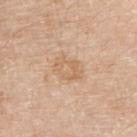subject: male, aged 78–82 | illumination: white-light illumination | location: the right upper arm | image: total-body-photography crop, ~15 mm field of view.